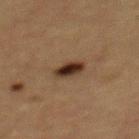workup: imaged on a skin check; not biopsied
patient: female, roughly 40 years of age
tile lighting: cross-polarized
automated metrics: a within-lesion color-variation index near 4.5/10 and radial color variation of about 1.5; a nevus-likeness score of about 100/100 and a detector confidence of about 100 out of 100 that the crop contains a lesion
site: the back
diameter: ~2.5 mm (longest diameter)
image source: ~15 mm tile from a whole-body skin photo